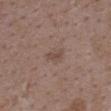Recorded during total-body skin imaging; not selected for excision or biopsy.
Automated image analysis of the tile measured an eccentricity of roughly 0.85. And it measured an average lesion color of about L≈47 a*≈16 b*≈24 (CIELAB), about 7 CIELAB-L* units darker than the surrounding skin, and a normalized border contrast of about 5.5.
Located on the back.
A female patient aged 33 to 37.
A 15 mm close-up tile from a total-body photography series done for melanoma screening.
About 2.5 mm across.